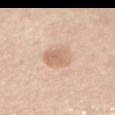The lesion was tiled from a total-body skin photograph and was not biopsied. From the abdomen. A region of skin cropped from a whole-body photographic capture, roughly 15 mm wide. The subject is a female aged 63 to 67. Automated image analysis of the tile measured a lesion area of about 8.5 mm², an eccentricity of roughly 0.7, and two-axis asymmetry of about 0.2. It also reported a lesion color around L≈67 a*≈18 b*≈31 in CIELAB and roughly 9 lightness units darker than nearby skin. And it measured a border-irregularity rating of about 2/10, internal color variation of about 3 on a 0–10 scale, and peripheral color asymmetry of about 1. And it measured a classifier nevus-likeness of about 20/100 and a lesion-detection confidence of about 100/100. This is a white-light tile.